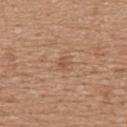biopsy status = total-body-photography surveillance lesion; no biopsy
site = the upper back
image = ~15 mm crop, total-body skin-cancer survey
subject = female, aged 43–47